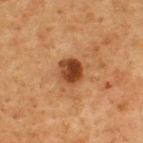Background: A 15 mm close-up extracted from a 3D total-body photography capture. Located on the upper back. An algorithmic analysis of the crop reported a mean CIELAB color near L≈34 a*≈21 b*≈31 and roughly 13 lightness units darker than nearby skin. And it measured a border-irregularity index near 1.5/10 and peripheral color asymmetry of about 2. And it measured a lesion-detection confidence of about 100/100. The patient is a male aged approximately 75. Imaged with cross-polarized lighting. Approximately 3 mm at its widest.